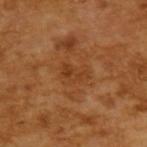Q: Is there a histopathology result?
A: imaged on a skin check; not biopsied
Q: How large is the lesion?
A: ≈3 mm
Q: How was the tile lit?
A: cross-polarized
Q: Automated lesion metrics?
A: an automated nevus-likeness rating near 0 out of 100 and a lesion-detection confidence of about 100/100
Q: Who is the patient?
A: male, about 65 years old
Q: How was this image acquired?
A: 15 mm crop, total-body photography